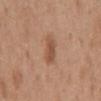workup=total-body-photography surveillance lesion; no biopsy
TBP lesion metrics=a mean CIELAB color near L≈52 a*≈21 b*≈32 and a lesion–skin lightness drop of about 9; a color-variation rating of about 2/10 and radial color variation of about 0.5
location=the mid back
lesion size=about 4 mm
subject=female, roughly 40 years of age
acquisition=~15 mm tile from a whole-body skin photo
illumination=white-light